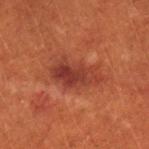A female patient in their 80s. About 6 mm across. A roughly 15 mm field-of-view crop from a total-body skin photograph. The lesion is on the left lower leg.